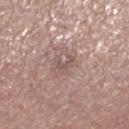workup — imaged on a skin check; not biopsied
size — ≈2.5 mm
patient — male, about 80 years old
acquisition — ~15 mm tile from a whole-body skin photo
illumination — white-light illumination
location — the left leg